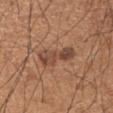No biopsy was performed on this lesion — it was imaged during a full skin examination and was not determined to be concerning. The tile uses white-light illumination. A region of skin cropped from a whole-body photographic capture, roughly 15 mm wide. Located on the left upper arm. A male patient approximately 65 years of age. Automated image analysis of the tile measured an area of roughly 7.5 mm² and a symmetry-axis asymmetry near 0.35. It also reported border irregularity of about 5.5 on a 0–10 scale and peripheral color asymmetry of about 1.5. The software also gave a nevus-likeness score of about 25/100 and lesion-presence confidence of about 100/100. The lesion's longest dimension is about 5 mm.